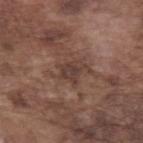Imaged during a routine full-body skin examination; the lesion was not biopsied and no histopathology is available.
A close-up tile cropped from a whole-body skin photograph, about 15 mm across.
On the left forearm.
Longest diameter approximately 3 mm.
A male patient approximately 75 years of age.
Automated image analysis of the tile measured a lesion color around L≈39 a*≈17 b*≈22 in CIELAB, about 7 CIELAB-L* units darker than the surrounding skin, and a lesion-to-skin contrast of about 6.5 (normalized; higher = more distinct). It also reported a border-irregularity rating of about 4.5/10 and radial color variation of about 0.5. It also reported a nevus-likeness score of about 0/100.